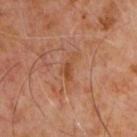Case summary:
– notes — catalogued during a skin exam; not biopsied
– tile lighting — cross-polarized illumination
– lesion size — ≈3.5 mm
– subject — male, about 60 years old
– image source — total-body-photography crop, ~15 mm field of view
– body site — the chest